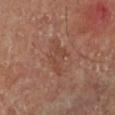Imaged during a routine full-body skin examination; the lesion was not biopsied and no histopathology is available. On the left lower leg. A 15 mm close-up tile from a total-body photography series done for melanoma screening. A male subject, approximately 65 years of age.